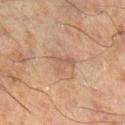{
  "biopsy_status": "not biopsied; imaged during a skin examination",
  "image": {
    "source": "total-body photography crop",
    "field_of_view_mm": 15
  },
  "lighting": "cross-polarized",
  "lesion_size": {
    "long_diameter_mm_approx": 3.0
  },
  "automated_metrics": {
    "border_irregularity_0_10": 5.5,
    "color_variation_0_10": 2.5,
    "peripheral_color_asymmetry": 1.0
  },
  "patient": {
    "sex": "male",
    "age_approx": 60
  },
  "site": "leg"
}